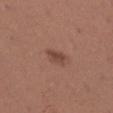Impression:
The lesion was tiled from a total-body skin photograph and was not biopsied.
Context:
The lesion is on the right lower leg. Captured under white-light illumination. This image is a 15 mm lesion crop taken from a total-body photograph. A female subject aged 23 to 27. The total-body-photography lesion software estimated a lesion area of about 3.5 mm² and an eccentricity of roughly 0.85. The software also gave border irregularity of about 3 on a 0–10 scale, internal color variation of about 2 on a 0–10 scale, and a peripheral color-asymmetry measure near 0.5. The analysis additionally found an automated nevus-likeness rating near 80 out of 100 and lesion-presence confidence of about 100/100. About 3 mm across.